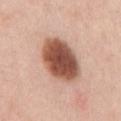Q: Is there a histopathology result?
A: no biopsy performed (imaged during a skin exam)
Q: What is the lesion's diameter?
A: ~6 mm (longest diameter)
Q: What kind of image is this?
A: ~15 mm crop, total-body skin-cancer survey
Q: Patient demographics?
A: male, about 50 years old
Q: Lesion location?
A: the chest
Q: Automated lesion metrics?
A: an outline eccentricity of about 0.7 (0 = round, 1 = elongated) and a symmetry-axis asymmetry near 0.1; a lesion color around L≈52 a*≈23 b*≈29 in CIELAB; internal color variation of about 5.5 on a 0–10 scale